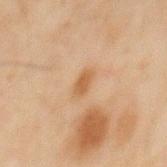follow-up: catalogued during a skin exam; not biopsied
location: the mid back
imaging modality: 15 mm crop, total-body photography
size: about 2.5 mm
subject: male, approximately 45 years of age